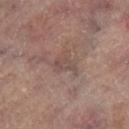Impression: The lesion was tiled from a total-body skin photograph and was not biopsied. Clinical summary: Imaged with cross-polarized lighting. The subject is a female in their 80s. From the left leg. Longest diameter approximately 2.5 mm. A roughly 15 mm field-of-view crop from a total-body skin photograph.